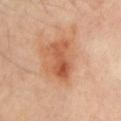• notes: imaged on a skin check; not biopsied
• anatomic site: the front of the torso
• diameter: ≈4.5 mm
• image: total-body-photography crop, ~15 mm field of view
• image-analysis metrics: an area of roughly 8.5 mm², a shape eccentricity near 0.85, and a symmetry-axis asymmetry near 0.3; a mean CIELAB color near L≈56 a*≈28 b*≈37, a lesion–skin lightness drop of about 11, and a lesion-to-skin contrast of about 7.5 (normalized; higher = more distinct); a border-irregularity index near 4/10, a color-variation rating of about 6.5/10, and radial color variation of about 2.5; a detector confidence of about 100 out of 100 that the crop contains a lesion